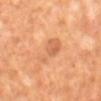On the mid back. The subject is a male aged 63–67. Longest diameter approximately 4.5 mm. A lesion tile, about 15 mm wide, cut from a 3D total-body photograph. An algorithmic analysis of the crop reported an outline eccentricity of about 0.85 (0 = round, 1 = elongated). The analysis additionally found a lesion-detection confidence of about 100/100. This is a cross-polarized tile.